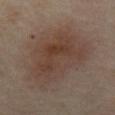Measured at roughly 8.5 mm in maximum diameter.
A close-up tile cropped from a whole-body skin photograph, about 15 mm across.
On the abdomen.
A female patient aged 48–52.
The total-body-photography lesion software estimated an eccentricity of roughly 0.7 and two-axis asymmetry of about 0.3. The analysis additionally found an automated nevus-likeness rating near 45 out of 100.